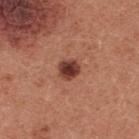workup = no biopsy performed (imaged during a skin exam)
body site = the front of the torso
patient = female, about 35 years old
lighting = white-light illumination
automated metrics = an area of roughly 5 mm², a shape eccentricity near 0.4, and two-axis asymmetry of about 0.25
acquisition = ~15 mm tile from a whole-body skin photo